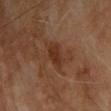Recorded during total-body skin imaging; not selected for excision or biopsy. The patient is a male in their 70s. From the upper back. A lesion tile, about 15 mm wide, cut from a 3D total-body photograph.